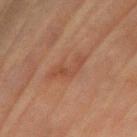Located on the left upper arm. A female subject, aged approximately 80. A close-up tile cropped from a whole-body skin photograph, about 15 mm across.